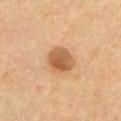Captured during whole-body skin photography for melanoma surveillance; the lesion was not biopsied.
A 15 mm close-up tile from a total-body photography series done for melanoma screening.
The lesion-visualizer software estimated a classifier nevus-likeness of about 90/100 and a lesion-detection confidence of about 100/100.
A male patient, aged 63–67.
The lesion is located on the upper back.
Approximately 3.5 mm at its widest.
This is a cross-polarized tile.